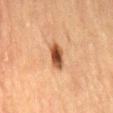The lesion-visualizer software estimated a mean CIELAB color near L≈46 a*≈22 b*≈34, roughly 16 lightness units darker than nearby skin, and a lesion-to-skin contrast of about 11.5 (normalized; higher = more distinct). And it measured a border-irregularity rating of about 1.5/10, internal color variation of about 5.5 on a 0–10 scale, and radial color variation of about 1.5. The analysis additionally found a classifier nevus-likeness of about 95/100. This is a cross-polarized tile. A region of skin cropped from a whole-body photographic capture, roughly 15 mm wide. The patient is a male roughly 85 years of age or older. The lesion is located on the mid back. About 3 mm across.